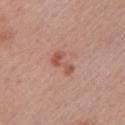| feature | finding |
|---|---|
| biopsy status | catalogued during a skin exam; not biopsied |
| patient | female, in their mid- to late 50s |
| imaging modality | 15 mm crop, total-body photography |
| location | the left upper arm |
| size | about 3.5 mm |